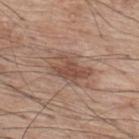Recorded during total-body skin imaging; not selected for excision or biopsy.
From the upper back.
Cropped from a whole-body photographic skin survey; the tile spans about 15 mm.
The subject is a male aged 68 to 72.
This is a white-light tile.
Measured at roughly 4.5 mm in maximum diameter.